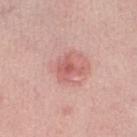Impression: This lesion was catalogued during total-body skin photography and was not selected for biopsy. Context: A roughly 15 mm field-of-view crop from a total-body skin photograph. The lesion's longest dimension is about 3 mm. Automated tile analysis of the lesion measured a lesion area of about 5.5 mm² and an eccentricity of roughly 0.35. It also reported internal color variation of about 3 on a 0–10 scale and radial color variation of about 0.5. And it measured a classifier nevus-likeness of about 5/100 and lesion-presence confidence of about 100/100. This is a white-light tile. On the right lower leg. A female subject in their mid- to late 40s.